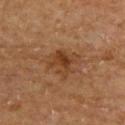Part of a total-body skin-imaging series; this lesion was reviewed on a skin check and was not flagged for biopsy. On the back. A 15 mm close-up extracted from a 3D total-body photography capture. The patient is a male in their mid-60s.